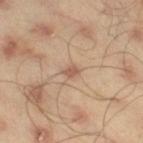Imaged during a routine full-body skin examination; the lesion was not biopsied and no histopathology is available. On the right thigh. A male patient aged 43 to 47. Automated image analysis of the tile measured a lesion area of about 2 mm² and two-axis asymmetry of about 0.25. The software also gave a mean CIELAB color near L≈55 a*≈19 b*≈29, about 10 CIELAB-L* units darker than the surrounding skin, and a normalized border contrast of about 6.5. It also reported a border-irregularity rating of about 2/10, a within-lesion color-variation index near 0.5/10, and radial color variation of about 0. The analysis additionally found a detector confidence of about 100 out of 100 that the crop contains a lesion. The tile uses cross-polarized illumination. The recorded lesion diameter is about 1.5 mm. Cropped from a total-body skin-imaging series; the visible field is about 15 mm.